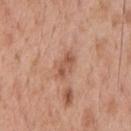The lesion was tiled from a total-body skin photograph and was not biopsied. The subject is a male approximately 55 years of age. The tile uses white-light illumination. A 15 mm crop from a total-body photograph taken for skin-cancer surveillance. Located on the chest. Measured at roughly 3.5 mm in maximum diameter.